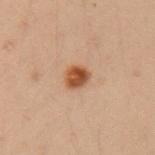workup: catalogued during a skin exam; not biopsied | site: the left upper arm | imaging modality: ~15 mm tile from a whole-body skin photo | subject: female, about 40 years old.